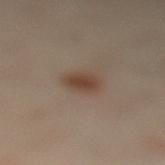Notes:
• notes: imaged on a skin check; not biopsied
• subject: female, aged 58 to 62
• body site: the right leg
• acquisition: ~15 mm crop, total-body skin-cancer survey